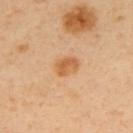Findings:
– follow-up · imaged on a skin check; not biopsied
– subject · male, about 40 years old
– image · ~15 mm tile from a whole-body skin photo
– automated metrics · a lesion–skin lightness drop of about 10 and a normalized border contrast of about 7.5; a border-irregularity index near 1.5/10, a within-lesion color-variation index near 3/10, and radial color variation of about 1; a classifier nevus-likeness of about 95/100 and a detector confidence of about 100 out of 100 that the crop contains a lesion
– site · the upper back
– lesion diameter · about 3 mm
– lighting · cross-polarized